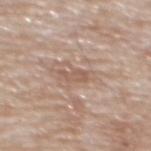The lesion was tiled from a total-body skin photograph and was not biopsied. A 15 mm close-up extracted from a 3D total-body photography capture. Approximately 3 mm at its widest. This is a white-light tile. A male patient roughly 80 years of age. On the mid back.